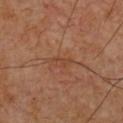The lesion was photographed on a routine skin check and not biopsied; there is no pathology result. On the chest. Longest diameter approximately 2.5 mm. The total-body-photography lesion software estimated an outline eccentricity of about 0.9 (0 = round, 1 = elongated) and a symmetry-axis asymmetry near 0.4. The analysis additionally found a lesion–skin lightness drop of about 5 and a normalized border contrast of about 4.5. The analysis additionally found an automated nevus-likeness rating near 0 out of 100 and a lesion-detection confidence of about 100/100. The subject is a male in their 60s. Imaged with cross-polarized lighting. A lesion tile, about 15 mm wide, cut from a 3D total-body photograph.